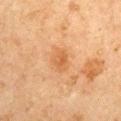No biopsy was performed on this lesion — it was imaged during a full skin examination and was not determined to be concerning. Automated tile analysis of the lesion measured a footprint of about 6.5 mm² and a shape-asymmetry score of about 0.2 (0 = symmetric). The software also gave a lesion color around L≈50 a*≈20 b*≈35 in CIELAB, about 7 CIELAB-L* units darker than the surrounding skin, and a normalized lesion–skin contrast near 5.5. The analysis additionally found border irregularity of about 1.5 on a 0–10 scale, internal color variation of about 3 on a 0–10 scale, and radial color variation of about 1. The lesion is on the left upper arm. The lesion's longest dimension is about 3.5 mm. The tile uses cross-polarized illumination. A male patient, roughly 65 years of age. A 15 mm close-up extracted from a 3D total-body photography capture.